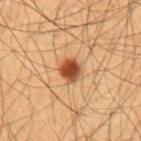Imaged during a routine full-body skin examination; the lesion was not biopsied and no histopathology is available.
About 2.5 mm across.
Captured under cross-polarized illumination.
An algorithmic analysis of the crop reported a lesion area of about 5.5 mm². The analysis additionally found lesion-presence confidence of about 100/100.
On the abdomen.
The subject is a male aged 53–57.
A close-up tile cropped from a whole-body skin photograph, about 15 mm across.